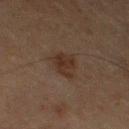workup: no biopsy performed (imaged during a skin exam).